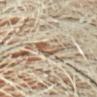biopsy status: total-body-photography surveillance lesion; no biopsy | image source: 15 mm crop, total-body photography | body site: the chest | patient: male, aged around 65 | lesion diameter: ≈4 mm | tile lighting: cross-polarized illumination.